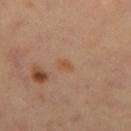On the left thigh.
A female subject aged 33–37.
The lesion's longest dimension is about 1.5 mm.
A close-up tile cropped from a whole-body skin photograph, about 15 mm across.
Imaged with cross-polarized lighting.